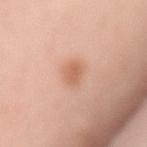From the mid back. Automated image analysis of the tile measured an area of roughly 4 mm², a shape eccentricity near 0.8, and a shape-asymmetry score of about 0.25 (0 = symmetric). The analysis additionally found an average lesion color of about L≈63 a*≈24 b*≈33 (CIELAB), about 9 CIELAB-L* units darker than the surrounding skin, and a normalized lesion–skin contrast near 6.5. About 3 mm across. The subject is a female aged approximately 50. A lesion tile, about 15 mm wide, cut from a 3D total-body photograph.